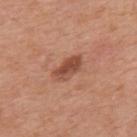automated metrics = a footprint of about 6 mm² and a symmetry-axis asymmetry near 0.15; border irregularity of about 2 on a 0–10 scale, a within-lesion color-variation index near 3.5/10, and a peripheral color-asymmetry measure near 1; an automated nevus-likeness rating near 70 out of 100 and a detector confidence of about 100 out of 100 that the crop contains a lesion | body site = the mid back | lighting = white-light | size = ~4 mm (longest diameter) | image source = ~15 mm crop, total-body skin-cancer survey | subject = male, aged 68 to 72.